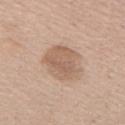<lesion>
  <biopsy_status>not biopsied; imaged during a skin examination</biopsy_status>
  <site>chest</site>
  <patient>
    <sex>male</sex>
    <age_approx>75</age_approx>
  </patient>
  <lighting>white-light</lighting>
  <image>
    <source>total-body photography crop</source>
    <field_of_view_mm>15</field_of_view_mm>
  </image>
</lesion>